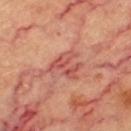Assessment: The lesion was tiled from a total-body skin photograph and was not biopsied. Acquisition and patient details: A female subject aged approximately 60. The lesion is located on the left thigh. A close-up tile cropped from a whole-body skin photograph, about 15 mm across. Measured at roughly 5 mm in maximum diameter. Imaged with cross-polarized lighting. The lesion-visualizer software estimated a footprint of about 9 mm², an eccentricity of roughly 0.65, and a shape-asymmetry score of about 0.5 (0 = symmetric). The analysis additionally found a mean CIELAB color near L≈56 a*≈32 b*≈29, a lesion–skin lightness drop of about 10, and a lesion-to-skin contrast of about 6.5 (normalized; higher = more distinct). And it measured a border-irregularity index near 7.5/10 and a color-variation rating of about 5.5/10. The analysis additionally found a nevus-likeness score of about 0/100 and lesion-presence confidence of about 95/100.